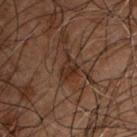Located on the chest. The lesion's longest dimension is about 3.5 mm. A close-up tile cropped from a whole-body skin photograph, about 15 mm across. The tile uses cross-polarized illumination. Automated image analysis of the tile measured a shape-asymmetry score of about 0.45 (0 = symmetric). The software also gave a lesion color around L≈22 a*≈14 b*≈20 in CIELAB, roughly 5 lightness units darker than nearby skin, and a normalized lesion–skin contrast near 6.5. The patient is a male in their 50s.